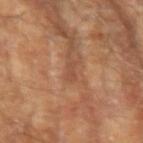Impression:
No biopsy was performed on this lesion — it was imaged during a full skin examination and was not determined to be concerning.
Background:
The lesion-visualizer software estimated an average lesion color of about L≈47 a*≈22 b*≈31 (CIELAB), roughly 7 lightness units darker than nearby skin, and a normalized lesion–skin contrast near 5.5. A region of skin cropped from a whole-body photographic capture, roughly 15 mm wide. The subject is a male in their mid-60s. From the left upper arm.